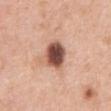This lesion was catalogued during total-body skin photography and was not selected for biopsy.
Captured under white-light illumination.
Approximately 3.5 mm at its widest.
A close-up tile cropped from a whole-body skin photograph, about 15 mm across.
The subject is a female approximately 40 years of age.
The lesion is on the chest.
The total-body-photography lesion software estimated border irregularity of about 1.5 on a 0–10 scale.